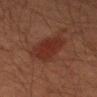Part of a total-body skin-imaging series; this lesion was reviewed on a skin check and was not flagged for biopsy.
Cropped from a whole-body photographic skin survey; the tile spans about 15 mm.
The lesion is on the right upper arm.
A male subject, in their mid- to late 40s.